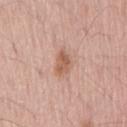biopsy status: imaged on a skin check; not biopsied | imaging modality: 15 mm crop, total-body photography | lesion size: about 3 mm | subject: male, about 60 years old | location: the lower back | lighting: white-light.